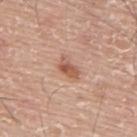Assessment:
Captured during whole-body skin photography for melanoma surveillance; the lesion was not biopsied.
Background:
From the back. A 15 mm crop from a total-body photograph taken for skin-cancer surveillance. The tile uses white-light illumination. The patient is a male aged around 75.